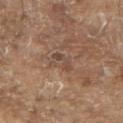No biopsy was performed on this lesion — it was imaged during a full skin examination and was not determined to be concerning.
Approximately 3 mm at its widest.
A male subject approximately 80 years of age.
The lesion is on the upper back.
A 15 mm close-up tile from a total-body photography series done for melanoma screening.
Automated image analysis of the tile measured an area of roughly 4.5 mm², a shape eccentricity near 0.8, and a shape-asymmetry score of about 0.4 (0 = symmetric). And it measured a mean CIELAB color near L≈47 a*≈18 b*≈26, a lesion–skin lightness drop of about 7, and a normalized border contrast of about 6. It also reported lesion-presence confidence of about 65/100.